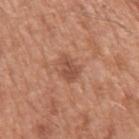Context: A lesion tile, about 15 mm wide, cut from a 3D total-body photograph. A male subject aged approximately 65. Approximately 3 mm at its widest. Imaged with white-light lighting. The lesion is located on the left upper arm.